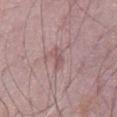follow-up: catalogued during a skin exam; not biopsied
image source: ~15 mm tile from a whole-body skin photo
diameter: ~3 mm (longest diameter)
site: the abdomen
subject: male, aged 63–67